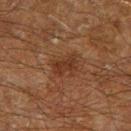Clinical impression:
The lesion was tiled from a total-body skin photograph and was not biopsied.
Image and clinical context:
A region of skin cropped from a whole-body photographic capture, roughly 15 mm wide. The lesion is on the right lower leg. A male patient roughly 60 years of age.